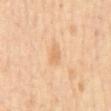Imaged during a routine full-body skin examination; the lesion was not biopsied and no histopathology is available. A female patient approximately 65 years of age. Located on the mid back. A 15 mm close-up extracted from a 3D total-body photography capture.